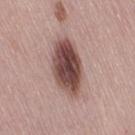Clinical summary: Automated tile analysis of the lesion measured an outline eccentricity of about 0.85 (0 = round, 1 = elongated) and a symmetry-axis asymmetry near 0.15. The software also gave a lesion color around L≈47 a*≈20 b*≈21 in CIELAB, roughly 18 lightness units darker than nearby skin, and a normalized border contrast of about 12.5. And it measured a nevus-likeness score of about 95/100 and lesion-presence confidence of about 100/100. On the right thigh. The recorded lesion diameter is about 7 mm. The tile uses white-light illumination. A lesion tile, about 15 mm wide, cut from a 3D total-body photograph. A female patient, aged around 50.